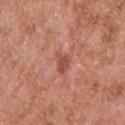{"biopsy_status": "not biopsied; imaged during a skin examination", "site": "upper back", "image": {"source": "total-body photography crop", "field_of_view_mm": 15}, "patient": {"sex": "male", "age_approx": 55}, "lesion_size": {"long_diameter_mm_approx": 2.5}, "automated_metrics": {"area_mm2_approx": 3.5, "eccentricity": 0.75, "shape_asymmetry": 0.4, "cielab_L": 50, "cielab_a": 27, "cielab_b": 29, "vs_skin_darker_L": 10.0, "vs_skin_contrast_norm": 7.0}, "lighting": "white-light"}